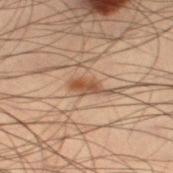<case>
  <biopsy_status>not biopsied; imaged during a skin examination</biopsy_status>
  <lighting>cross-polarized</lighting>
  <lesion_size>
    <long_diameter_mm_approx>3.5</long_diameter_mm_approx>
  </lesion_size>
  <site>left thigh</site>
  <image>
    <source>total-body photography crop</source>
    <field_of_view_mm>15</field_of_view_mm>
  </image>
  <patient>
    <sex>male</sex>
    <age_approx>55</age_approx>
  </patient>
  <automated_metrics>
    <area_mm2_approx>4.5</area_mm2_approx>
    <shape_asymmetry>0.25</shape_asymmetry>
    <vs_skin_darker_L>9.0</vs_skin_darker_L>
    <peripheral_color_asymmetry>1.0</peripheral_color_asymmetry>
    <nevus_likeness_0_100>70</nevus_likeness_0_100>
    <lesion_detection_confidence_0_100>100</lesion_detection_confidence_0_100>
  </automated_metrics>
</case>